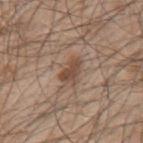notes — total-body-photography surveillance lesion; no biopsy | image source — ~15 mm crop, total-body skin-cancer survey | patient — male, aged around 50 | body site — the left upper arm | lesion diameter — ≈3.5 mm | image-analysis metrics — a mean CIELAB color near L≈48 a*≈17 b*≈27 and a normalized lesion–skin contrast near 7.5; a border-irregularity rating of about 4/10 and a peripheral color-asymmetry measure near 1; a detector confidence of about 100 out of 100 that the crop contains a lesion.